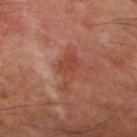  biopsy_status: not biopsied; imaged during a skin examination
  lighting: cross-polarized
  lesion_size:
    long_diameter_mm_approx: 4.0
  patient:
    sex: male
    age_approx: 70
  image:
    source: total-body photography crop
    field_of_view_mm: 15
  site: left thigh